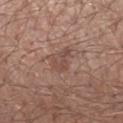A male patient in their 60s. A close-up tile cropped from a whole-body skin photograph, about 15 mm across. An algorithmic analysis of the crop reported an area of roughly 4.5 mm², an eccentricity of roughly 0.75, and two-axis asymmetry of about 0.5. Longest diameter approximately 3 mm. The lesion is on the left forearm.